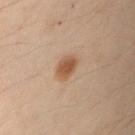Located on the left upper arm. A 15 mm crop from a total-body photograph taken for skin-cancer surveillance. A male subject aged approximately 50.